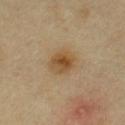Assessment:
Captured during whole-body skin photography for melanoma surveillance; the lesion was not biopsied.
Image and clinical context:
The lesion is on the chest. Automated image analysis of the tile measured a shape eccentricity near 0.4 and a symmetry-axis asymmetry near 0.1. It also reported about 10 CIELAB-L* units darker than the surrounding skin and a normalized border contrast of about 8.5. And it measured a border-irregularity index near 1.5/10, a within-lesion color-variation index near 6.5/10, and radial color variation of about 2.5. The tile uses cross-polarized illumination. The subject is a female aged around 50. Longest diameter approximately 3 mm. This image is a 15 mm lesion crop taken from a total-body photograph.